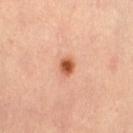biopsy status — total-body-photography surveillance lesion; no biopsy
imaging modality — ~15 mm crop, total-body skin-cancer survey
subject — female, aged 28 to 32
location — the left thigh